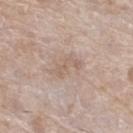biopsy status: no biopsy performed (imaged during a skin exam) | diameter: ~4 mm (longest diameter) | subject: female, in their mid- to late 70s | location: the left lower leg | TBP lesion metrics: a mean CIELAB color near L≈61 a*≈15 b*≈26, a lesion–skin lightness drop of about 7, and a lesion-to-skin contrast of about 5 (normalized; higher = more distinct); border irregularity of about 5 on a 0–10 scale and radial color variation of about 0.5 | image source: ~15 mm crop, total-body skin-cancer survey.